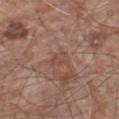biopsy status=total-body-photography surveillance lesion; no biopsy
TBP lesion metrics=about 6 CIELAB-L* units darker than the surrounding skin; a border-irregularity rating of about 2.5/10 and peripheral color asymmetry of about 0.5; lesion-presence confidence of about 95/100
lighting=white-light illumination
size=~2.5 mm (longest diameter)
body site=the chest
subject=male, roughly 65 years of age
image=~15 mm crop, total-body skin-cancer survey